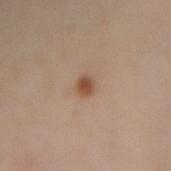notes — no biopsy performed (imaged during a skin exam)
diameter — ~2 mm (longest diameter)
body site — the right forearm
imaging modality — ~15 mm crop, total-body skin-cancer survey
illumination — cross-polarized illumination
automated metrics — a lesion area of about 3 mm² and a shape-asymmetry score of about 0.4 (0 = symmetric); a mean CIELAB color near L≈49 a*≈19 b*≈31, about 11 CIELAB-L* units darker than the surrounding skin, and a lesion-to-skin contrast of about 8.5 (normalized; higher = more distinct); a border-irregularity index near 3/10 and a peripheral color-asymmetry measure near 0.5; a nevus-likeness score of about 100/100 and a lesion-detection confidence of about 100/100
subject — female, aged 38–42